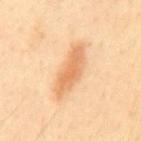Recorded during total-body skin imaging; not selected for excision or biopsy. The recorded lesion diameter is about 7 mm. A male patient, aged 48–52. Imaged with cross-polarized lighting. A 15 mm crop from a total-body photograph taken for skin-cancer surveillance. From the mid back.